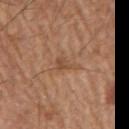{"biopsy_status": "not biopsied; imaged during a skin examination", "image": {"source": "total-body photography crop", "field_of_view_mm": 15}, "automated_metrics": {"eccentricity": 0.85, "shape_asymmetry": 0.5}, "site": "arm", "lighting": "white-light", "lesion_size": {"long_diameter_mm_approx": 3.0}, "patient": {"sex": "male", "age_approx": 65}}